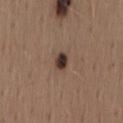{"biopsy_status": "not biopsied; imaged during a skin examination"}